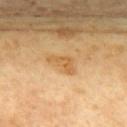Part of a total-body skin-imaging series; this lesion was reviewed on a skin check and was not flagged for biopsy.
The lesion is on the front of the torso.
Automated image analysis of the tile measured an average lesion color of about L≈62 a*≈20 b*≈44 (CIELAB), a lesion–skin lightness drop of about 9, and a normalized lesion–skin contrast near 6.5.
Measured at roughly 3.5 mm in maximum diameter.
A close-up tile cropped from a whole-body skin photograph, about 15 mm across.
A female patient, aged 73 to 77.